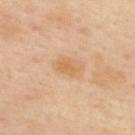• workup · catalogued during a skin exam; not biopsied
• automated metrics · an area of roughly 3.5 mm², a shape eccentricity near 0.8, and a shape-asymmetry score of about 0.25 (0 = symmetric); a border-irregularity rating of about 2.5/10, a within-lesion color-variation index near 1/10, and a peripheral color-asymmetry measure near 0.5
• body site · the upper back
• diameter · about 3 mm
• image · 15 mm crop, total-body photography
• tile lighting · cross-polarized
• subject · female, aged approximately 40